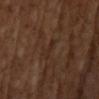A 15 mm close-up extracted from a 3D total-body photography capture. Approximately 3 mm at its widest. Imaged with cross-polarized lighting. The subject is a male aged approximately 65.